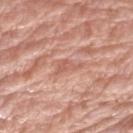follow-up — no biopsy performed (imaged during a skin exam) | image-analysis metrics — an area of roughly 3.5 mm², an outline eccentricity of about 0.85 (0 = round, 1 = elongated), and a shape-asymmetry score of about 0.55 (0 = symmetric); a border-irregularity rating of about 5.5/10, a within-lesion color-variation index near 1/10, and peripheral color asymmetry of about 0.5 | patient — male, in their 80s | image — ~15 mm crop, total-body skin-cancer survey | size — ≈3 mm | site — the left upper arm.